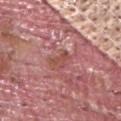notes: no biopsy performed (imaged during a skin exam) | tile lighting: white-light illumination | subject: male, about 40 years old | body site: the left upper arm | image-analysis metrics: a lesion area of about 4 mm², an outline eccentricity of about 0.7 (0 = round, 1 = elongated), and a shape-asymmetry score of about 0.5 (0 = symmetric); about 7 CIELAB-L* units darker than the surrounding skin and a normalized border contrast of about 6; lesion-presence confidence of about 75/100 | diameter: about 2.5 mm | image source: total-body-photography crop, ~15 mm field of view.